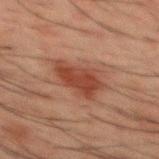workup: no biopsy performed (imaged during a skin exam)
size: ≈4.5 mm
image-analysis metrics: a footprint of about 9.5 mm² and a shape-asymmetry score of about 0.35 (0 = symmetric); internal color variation of about 2 on a 0–10 scale and peripheral color asymmetry of about 1
patient: male, in their 50s
image source: ~15 mm crop, total-body skin-cancer survey
tile lighting: cross-polarized illumination
anatomic site: the back